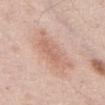Q: Is there a histopathology result?
A: imaged on a skin check; not biopsied
Q: What is the imaging modality?
A: total-body-photography crop, ~15 mm field of view
Q: What are the patient's age and sex?
A: male, in their mid- to late 50s
Q: What is the anatomic site?
A: the abdomen